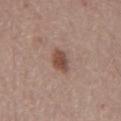A male subject in their 80s. Measured at roughly 3 mm in maximum diameter. Captured under white-light illumination. An algorithmic analysis of the crop reported a lesion area of about 5 mm², a shape eccentricity near 0.75, and a shape-asymmetry score of about 0.25 (0 = symmetric). The analysis additionally found a nevus-likeness score of about 85/100 and a detector confidence of about 100 out of 100 that the crop contains a lesion. A lesion tile, about 15 mm wide, cut from a 3D total-body photograph. The lesion is on the front of the torso.